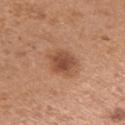{
  "patient": {
    "sex": "female",
    "age_approx": 30
  },
  "site": "left upper arm",
  "image": {
    "source": "total-body photography crop",
    "field_of_view_mm": 15
  }
}